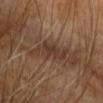Q: Is there a histopathology result?
A: total-body-photography surveillance lesion; no biopsy
Q: Automated lesion metrics?
A: a lesion area of about 9 mm², a shape eccentricity near 0.75, and a symmetry-axis asymmetry near 0.35; a classifier nevus-likeness of about 0/100
Q: Patient demographics?
A: male, aged 58–62
Q: Lesion location?
A: the right upper arm
Q: What kind of image is this?
A: ~15 mm tile from a whole-body skin photo
Q: What lighting was used for the tile?
A: cross-polarized
Q: How large is the lesion?
A: about 4 mm